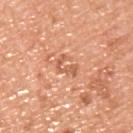Recorded during total-body skin imaging; not selected for excision or biopsy. Located on the upper back. The subject is a male aged 48–52. The tile uses white-light illumination. Cropped from a total-body skin-imaging series; the visible field is about 15 mm. The recorded lesion diameter is about 2.5 mm. Automated image analysis of the tile measured an area of roughly 3.5 mm², an eccentricity of roughly 0.75, and a shape-asymmetry score of about 0.55 (0 = symmetric). The software also gave an average lesion color of about L≈61 a*≈27 b*≈36 (CIELAB), about 9 CIELAB-L* units darker than the surrounding skin, and a normalized lesion–skin contrast near 5.5. The software also gave a border-irregularity rating of about 8/10 and internal color variation of about 0 on a 0–10 scale. The software also gave a classifier nevus-likeness of about 0/100 and lesion-presence confidence of about 100/100.